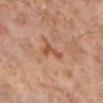The lesion was photographed on a routine skin check and not biopsied; there is no pathology result.
The subject is a male about 60 years old.
On the leg.
A lesion tile, about 15 mm wide, cut from a 3D total-body photograph.
This is a cross-polarized tile.
The recorded lesion diameter is about 3 mm.